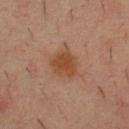biopsy_status: not biopsied; imaged during a skin examination
automated_metrics:
  area_mm2_approx: 7.5
  eccentricity: 0.45
  shape_asymmetry: 0.25
  nevus_likeness_0_100: 100
image:
  source: total-body photography crop
  field_of_view_mm: 15
patient:
  sex: male
  age_approx: 35
lesion_size:
  long_diameter_mm_approx: 3.0
lighting: cross-polarized
site: chest